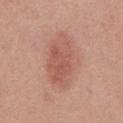Q: Was a biopsy performed?
A: catalogued during a skin exam; not biopsied
Q: Patient demographics?
A: male, aged around 30
Q: What kind of image is this?
A: ~15 mm crop, total-body skin-cancer survey
Q: What is the anatomic site?
A: the chest